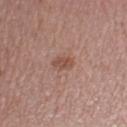Assessment: The lesion was tiled from a total-body skin photograph and was not biopsied. Acquisition and patient details: Located on the left upper arm. A male patient in their mid- to late 50s. The recorded lesion diameter is about 2.5 mm. The total-body-photography lesion software estimated a lesion area of about 4 mm², an eccentricity of roughly 0.75, and a shape-asymmetry score of about 0.25 (0 = symmetric). It also reported a border-irregularity index near 2/10, a color-variation rating of about 2/10, and peripheral color asymmetry of about 1. This is a white-light tile. Cropped from a total-body skin-imaging series; the visible field is about 15 mm.